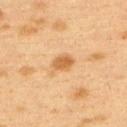biopsy status=catalogued during a skin exam; not biopsied | automated lesion analysis=a footprint of about 4.5 mm² and an eccentricity of roughly 0.7; an automated nevus-likeness rating near 90 out of 100 and lesion-presence confidence of about 100/100 | image=total-body-photography crop, ~15 mm field of view | lesion diameter=~2.5 mm (longest diameter) | illumination=cross-polarized illumination | subject=female, roughly 40 years of age | anatomic site=the upper back.